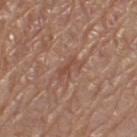Q: Was a biopsy performed?
A: no biopsy performed (imaged during a skin exam)
Q: Lesion size?
A: ~3.5 mm (longest diameter)
Q: How was this image acquired?
A: 15 mm crop, total-body photography
Q: What are the patient's age and sex?
A: female, approximately 55 years of age
Q: Automated lesion metrics?
A: an area of roughly 4.5 mm², an outline eccentricity of about 0.85 (0 = round, 1 = elongated), and two-axis asymmetry of about 0.35; a mean CIELAB color near L≈49 a*≈21 b*≈27, about 7 CIELAB-L* units darker than the surrounding skin, and a normalized lesion–skin contrast near 5.5; border irregularity of about 4.5 on a 0–10 scale, a color-variation rating of about 2/10, and peripheral color asymmetry of about 0.5; a classifier nevus-likeness of about 0/100 and a lesion-detection confidence of about 60/100
Q: Where on the body is the lesion?
A: the left thigh
Q: How was the tile lit?
A: white-light illumination